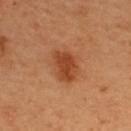Q: Was this lesion biopsied?
A: no biopsy performed (imaged during a skin exam)
Q: How was this image acquired?
A: ~15 mm tile from a whole-body skin photo
Q: Automated lesion metrics?
A: an average lesion color of about L≈43 a*≈27 b*≈36 (CIELAB) and a lesion-to-skin contrast of about 8 (normalized; higher = more distinct); a classifier nevus-likeness of about 95/100
Q: Patient demographics?
A: male, in their mid-30s
Q: How was the tile lit?
A: cross-polarized
Q: Lesion size?
A: ≈4 mm
Q: Lesion location?
A: the back